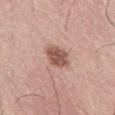{"biopsy_status": "not biopsied; imaged during a skin examination"}